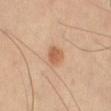automated metrics: an average lesion color of about L≈59 a*≈22 b*≈35 (CIELAB) and about 11 CIELAB-L* units darker than the surrounding skin; a nevus-likeness score of about 90/100 and a lesion-detection confidence of about 100/100 | image source: total-body-photography crop, ~15 mm field of view | lighting: cross-polarized illumination | patient: male, about 65 years old | anatomic site: the left thigh | size: ~2.5 mm (longest diameter).